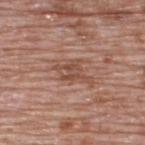Imaged during a routine full-body skin examination; the lesion was not biopsied and no histopathology is available. Approximately 4.5 mm at its widest. A male subject, aged approximately 70. A region of skin cropped from a whole-body photographic capture, roughly 15 mm wide. Located on the upper back. An algorithmic analysis of the crop reported a nevus-likeness score of about 0/100.